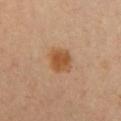Assessment: No biopsy was performed on this lesion — it was imaged during a full skin examination and was not determined to be concerning. Image and clinical context: A 15 mm crop from a total-body photograph taken for skin-cancer surveillance. The patient is a female about 30 years old. From the chest.